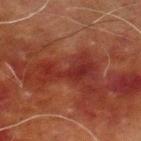| field | value |
|---|---|
| follow-up | no biopsy performed (imaged during a skin exam) |
| subject | male, aged 68 to 72 |
| site | the left lower leg |
| illumination | cross-polarized illumination |
| image | ~15 mm tile from a whole-body skin photo |
| lesion diameter | about 5.5 mm |